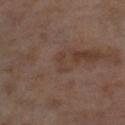Clinical impression:
Recorded during total-body skin imaging; not selected for excision or biopsy.